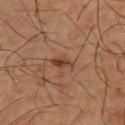The lesion was photographed on a routine skin check and not biopsied; there is no pathology result.
A male patient, about 65 years old.
From the leg.
A 15 mm close-up extracted from a 3D total-body photography capture.
Imaged with cross-polarized lighting.